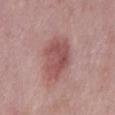Part of a total-body skin-imaging series; this lesion was reviewed on a skin check and was not flagged for biopsy.
A male patient, in their mid-50s.
A lesion tile, about 15 mm wide, cut from a 3D total-body photograph.
The lesion is on the mid back.